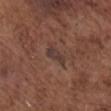Q: What is the anatomic site?
A: the head or neck
Q: What kind of image is this?
A: total-body-photography crop, ~15 mm field of view
Q: Who is the patient?
A: male, aged 73–77